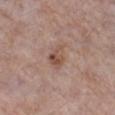No biopsy was performed on this lesion — it was imaged during a full skin examination and was not determined to be concerning.
A female patient approximately 70 years of age.
A 15 mm crop from a total-body photograph taken for skin-cancer surveillance.
The tile uses white-light illumination.
On the leg.
About 3 mm across.